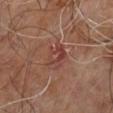follow-up: total-body-photography surveillance lesion; no biopsy | size: ~3.5 mm (longest diameter) | acquisition: 15 mm crop, total-body photography | patient: male, aged 58–62 | anatomic site: the right leg.